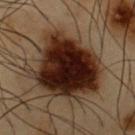Q: Is there a histopathology result?
A: total-body-photography surveillance lesion; no biopsy
Q: Who is the patient?
A: male, in their mid-50s
Q: What is the anatomic site?
A: the chest
Q: What is the imaging modality?
A: ~15 mm tile from a whole-body skin photo
Q: What lighting was used for the tile?
A: cross-polarized illumination
Q: Lesion size?
A: ≈8.5 mm
Q: Automated lesion metrics?
A: a border-irregularity rating of about 3/10 and internal color variation of about 8 on a 0–10 scale; a nevus-likeness score of about 100/100 and a detector confidence of about 100 out of 100 that the crop contains a lesion